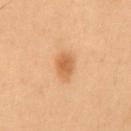<tbp_lesion>
  <biopsy_status>not biopsied; imaged during a skin examination</biopsy_status>
</tbp_lesion>